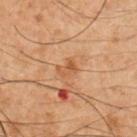follow-up: imaged on a skin check; not biopsied.